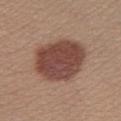Q: Is there a histopathology result?
A: total-body-photography surveillance lesion; no biopsy
Q: What lighting was used for the tile?
A: white-light
Q: Where on the body is the lesion?
A: the left lower leg
Q: What kind of image is this?
A: ~15 mm crop, total-body skin-cancer survey
Q: How large is the lesion?
A: ≈6.5 mm
Q: Who is the patient?
A: male, about 30 years old
Q: Automated lesion metrics?
A: a lesion-to-skin contrast of about 10.5 (normalized; higher = more distinct); a border-irregularity rating of about 1.5/10, a within-lesion color-variation index near 3.5/10, and a peripheral color-asymmetry measure near 1.5